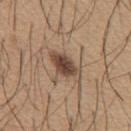Assessment:
Recorded during total-body skin imaging; not selected for excision or biopsy.
Clinical summary:
The lesion is on the chest. The lesion's longest dimension is about 4.5 mm. A male subject, aged approximately 65. A close-up tile cropped from a whole-body skin photograph, about 15 mm across. An algorithmic analysis of the crop reported a footprint of about 8 mm², a shape eccentricity near 0.85, and two-axis asymmetry of about 0.25. And it measured a lesion color around L≈46 a*≈17 b*≈27 in CIELAB, roughly 14 lightness units darker than nearby skin, and a normalized border contrast of about 10. The software also gave a nevus-likeness score of about 90/100. This is a white-light tile.